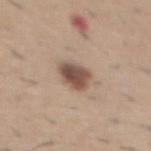biopsy status = total-body-photography surveillance lesion; no biopsy
illumination = white-light
acquisition = total-body-photography crop, ~15 mm field of view
automated metrics = a footprint of about 7.5 mm², a shape eccentricity near 0.55, and a symmetry-axis asymmetry near 0.2; a border-irregularity index near 2/10 and a within-lesion color-variation index near 4/10; a classifier nevus-likeness of about 85/100 and a lesion-detection confidence of about 100/100
patient = male, about 60 years old
location = the abdomen